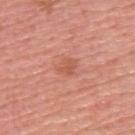Q: Was a biopsy performed?
A: imaged on a skin check; not biopsied
Q: What are the patient's age and sex?
A: male, aged 53 to 57
Q: What lighting was used for the tile?
A: white-light illumination
Q: What is the lesion's diameter?
A: ~3 mm (longest diameter)
Q: Lesion location?
A: the back
Q: What is the imaging modality?
A: ~15 mm tile from a whole-body skin photo
Q: What did automated image analysis measure?
A: a mean CIELAB color near L≈57 a*≈28 b*≈33, about 7 CIELAB-L* units darker than the surrounding skin, and a normalized border contrast of about 5.5; border irregularity of about 3 on a 0–10 scale, a within-lesion color-variation index near 1.5/10, and a peripheral color-asymmetry measure near 0.5